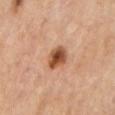Part of a total-body skin-imaging series; this lesion was reviewed on a skin check and was not flagged for biopsy.
The patient is a male roughly 70 years of age.
On the mid back.
The lesion's longest dimension is about 3 mm.
A lesion tile, about 15 mm wide, cut from a 3D total-body photograph.
Imaged with cross-polarized lighting.